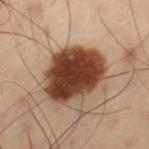Q: Was this lesion biopsied?
A: imaged on a skin check; not biopsied
Q: How was this image acquired?
A: total-body-photography crop, ~15 mm field of view
Q: Patient demographics?
A: male, approximately 55 years of age
Q: What did automated image analysis measure?
A: a footprint of about 27 mm², an outline eccentricity of about 0.6 (0 = round, 1 = elongated), and a shape-asymmetry score of about 0.15 (0 = symmetric); an average lesion color of about L≈31 a*≈19 b*≈24 (CIELAB), about 19 CIELAB-L* units darker than the surrounding skin, and a normalized lesion–skin contrast near 16
Q: Illumination type?
A: cross-polarized
Q: Where on the body is the lesion?
A: the left thigh
Q: Lesion size?
A: about 6.5 mm